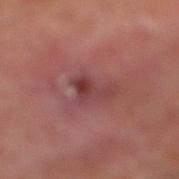Findings:
* follow-up · catalogued during a skin exam; not biopsied
* patient · male, roughly 70 years of age
* automated lesion analysis · an area of roughly 5 mm², an eccentricity of roughly 0.75, and a symmetry-axis asymmetry near 0.7; a lesion color around L≈39 a*≈26 b*≈20 in CIELAB, about 8 CIELAB-L* units darker than the surrounding skin, and a normalized lesion–skin contrast near 7; a within-lesion color-variation index near 3/10 and radial color variation of about 0.5; a classifier nevus-likeness of about 0/100
* illumination · cross-polarized illumination
* body site · the right lower leg
* lesion diameter · ≈3.5 mm
* imaging modality · total-body-photography crop, ~15 mm field of view